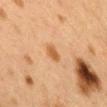{"biopsy_status": "not biopsied; imaged during a skin examination", "lesion_size": {"long_diameter_mm_approx": 2.5}, "patient": {"sex": "female", "age_approx": 40}, "site": "upper back", "automated_metrics": {"eccentricity": 0.8, "shape_asymmetry": 0.3, "vs_skin_contrast_norm": 7.5}, "lighting": "cross-polarized", "image": {"source": "total-body photography crop", "field_of_view_mm": 15}}